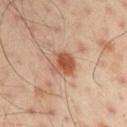Impression: This lesion was catalogued during total-body skin photography and was not selected for biopsy. Context: Captured under cross-polarized illumination. This image is a 15 mm lesion crop taken from a total-body photograph. The lesion is on the left arm. A male subject, in their 50s. The recorded lesion diameter is about 3 mm.